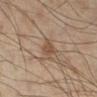<case>
  <biopsy_status>not biopsied; imaged during a skin examination</biopsy_status>
  <image>
    <source>total-body photography crop</source>
    <field_of_view_mm>15</field_of_view_mm>
  </image>
  <site>leg</site>
  <lighting>cross-polarized</lighting>
  <patient>
    <sex>male</sex>
    <age_approx>55</age_approx>
  </patient>
  <lesion_size>
    <long_diameter_mm_approx>2.0</long_diameter_mm_approx>
  </lesion_size>
</case>